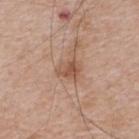Impression:
Captured during whole-body skin photography for melanoma surveillance; the lesion was not biopsied.
Clinical summary:
Captured under white-light illumination. The lesion is located on the upper back. The lesion's longest dimension is about 3 mm. A male subject aged approximately 65. Cropped from a total-body skin-imaging series; the visible field is about 15 mm.